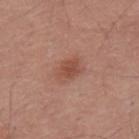<tbp_lesion>
<biopsy_status>not biopsied; imaged during a skin examination</biopsy_status>
<lesion_size>
  <long_diameter_mm_approx>3.0</long_diameter_mm_approx>
</lesion_size>
<patient>
  <sex>male</sex>
  <age_approx>40</age_approx>
</patient>
<automated_metrics>
  <eccentricity>0.7</eccentricity>
  <shape_asymmetry>0.25</shape_asymmetry>
</automated_metrics>
<site>back</site>
<image>
  <source>total-body photography crop</source>
  <field_of_view_mm>15</field_of_view_mm>
</image>
</tbp_lesion>